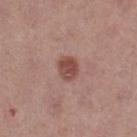body site = the leg
acquisition = total-body-photography crop, ~15 mm field of view
subject = female, approximately 40 years of age